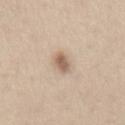workup: imaged on a skin check; not biopsied | site: the abdomen | TBP lesion metrics: an eccentricity of roughly 0.9 and a symmetry-axis asymmetry near 0.25; a mean CIELAB color near L≈61 a*≈15 b*≈28, about 12 CIELAB-L* units darker than the surrounding skin, and a lesion-to-skin contrast of about 8 (normalized; higher = more distinct); a border-irregularity index near 2.5/10, a color-variation rating of about 3.5/10, and radial color variation of about 1 | subject: female, aged 43–47 | size: ≈3.5 mm | image source: 15 mm crop, total-body photography.